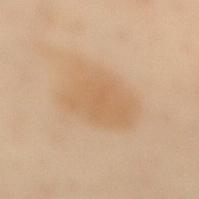Impression: This lesion was catalogued during total-body skin photography and was not selected for biopsy. Context: About 6.5 mm across. On the mid back. The subject is a female aged approximately 60. This image is a 15 mm lesion crop taken from a total-body photograph. Captured under cross-polarized illumination. An algorithmic analysis of the crop reported a footprint of about 23 mm² and a shape-asymmetry score of about 0.25 (0 = symmetric). And it measured a mean CIELAB color near L≈54 a*≈14 b*≈32 and a lesion-to-skin contrast of about 5.5 (normalized; higher = more distinct). The analysis additionally found an automated nevus-likeness rating near 10 out of 100.